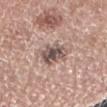image:
  source: total-body photography crop
  field_of_view_mm: 15
lighting: white-light
lesion_size:
  long_diameter_mm_approx: 3.5
automated_metrics:
  cielab_L: 52
  cielab_a: 15
  cielab_b: 20
  vs_skin_darker_L: 14.0
  vs_skin_contrast_norm: 9.5
patient:
  sex: female
  age_approx: 50
site: right forearm